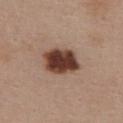biopsy status = catalogued during a skin exam; not biopsied
tile lighting = white-light illumination
automated lesion analysis = a lesion color around L≈40 a*≈20 b*≈25 in CIELAB, roughly 20 lightness units darker than nearby skin, and a normalized border contrast of about 14.5; a border-irregularity index near 2/10, a within-lesion color-variation index near 6/10, and radial color variation of about 2
subject = female, in their mid- to late 50s
image = 15 mm crop, total-body photography
size = ~5 mm (longest diameter)
anatomic site = the chest